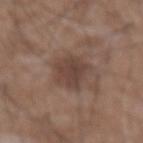Case summary:
• workup — total-body-photography surveillance lesion; no biopsy
• patient — male, about 75 years old
• anatomic site — the abdomen
• image source — 15 mm crop, total-body photography